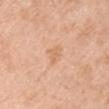Impression: Captured during whole-body skin photography for melanoma surveillance; the lesion was not biopsied. Image and clinical context: Captured under white-light illumination. A female patient, aged 43 to 47. On the right upper arm. Automated image analysis of the tile measured a lesion area of about 3.5 mm² and two-axis asymmetry of about 0.3. It also reported a border-irregularity index near 3/10, a color-variation rating of about 1/10, and radial color variation of about 0.5. A 15 mm close-up extracted from a 3D total-body photography capture.